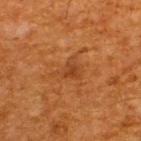A 15 mm crop from a total-body photograph taken for skin-cancer surveillance. The lesion's longest dimension is about 3.5 mm. A male subject roughly 65 years of age. The lesion-visualizer software estimated a footprint of about 5 mm², an outline eccentricity of about 0.65 (0 = round, 1 = elongated), and two-axis asymmetry of about 0.45. The software also gave a border-irregularity rating of about 5/10, a within-lesion color-variation index near 2/10, and peripheral color asymmetry of about 0.5. On the upper back.